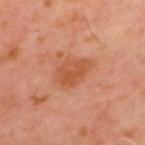Imaged during a routine full-body skin examination; the lesion was not biopsied and no histopathology is available. A male patient, approximately 60 years of age. A 15 mm crop from a total-body photograph taken for skin-cancer surveillance. From the mid back.